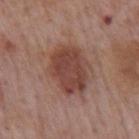Part of a total-body skin-imaging series; this lesion was reviewed on a skin check and was not flagged for biopsy.
From the mid back.
A male patient, in their mid- to late 70s.
Imaged with white-light lighting.
Cropped from a total-body skin-imaging series; the visible field is about 15 mm.
Automated image analysis of the tile measured a footprint of about 19 mm², a shape eccentricity near 0.7, and a symmetry-axis asymmetry near 0.15. The analysis additionally found a lesion color around L≈43 a*≈22 b*≈25 in CIELAB, roughly 11 lightness units darker than nearby skin, and a normalized lesion–skin contrast near 8.5. The analysis additionally found border irregularity of about 1.5 on a 0–10 scale, internal color variation of about 3 on a 0–10 scale, and a peripheral color-asymmetry measure near 1.
Approximately 6 mm at its widest.